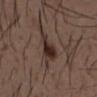Q: Is there a histopathology result?
A: imaged on a skin check; not biopsied
Q: How was this image acquired?
A: ~15 mm crop, total-body skin-cancer survey
Q: How was the tile lit?
A: white-light illumination
Q: How large is the lesion?
A: about 4 mm
Q: What did automated image analysis measure?
A: a lesion area of about 7.5 mm², an outline eccentricity of about 0.85 (0 = round, 1 = elongated), and two-axis asymmetry of about 0.4; a border-irregularity rating of about 4/10, a color-variation rating of about 4.5/10, and a peripheral color-asymmetry measure near 1.5; a nevus-likeness score of about 100/100
Q: Lesion location?
A: the front of the torso
Q: What are the patient's age and sex?
A: male, in their 50s